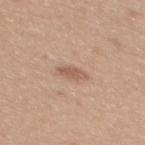biopsy status=catalogued during a skin exam; not biopsied
anatomic site=the mid back
size=about 3 mm
imaging modality=15 mm crop, total-body photography
tile lighting=white-light
patient=female, in their mid-30s